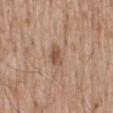The lesion was tiled from a total-body skin photograph and was not biopsied. On the back. The lesion's longest dimension is about 3.5 mm. Cropped from a whole-body photographic skin survey; the tile spans about 15 mm. The subject is a male aged 53 to 57. The tile uses white-light illumination.